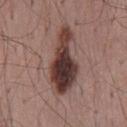Imaged during a routine full-body skin examination; the lesion was not biopsied and no histopathology is available. The lesion's longest dimension is about 8 mm. A male patient aged 48–52. From the mid back. Captured under white-light illumination. A 15 mm close-up tile from a total-body photography series done for melanoma screening. The lesion-visualizer software estimated a lesion area of about 20 mm², a shape eccentricity near 0.9, and two-axis asymmetry of about 0.45.